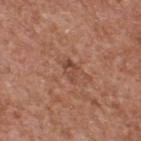Q: Was this lesion biopsied?
A: total-body-photography surveillance lesion; no biopsy
Q: What is the anatomic site?
A: the back
Q: How was this image acquired?
A: ~15 mm tile from a whole-body skin photo
Q: Who is the patient?
A: male, aged around 65